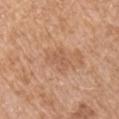Clinical impression: No biopsy was performed on this lesion — it was imaged during a full skin examination and was not determined to be concerning. Background: Captured under white-light illumination. Longest diameter approximately 3 mm. On the left upper arm. Cropped from a whole-body photographic skin survey; the tile spans about 15 mm. A male patient, aged 53–57.